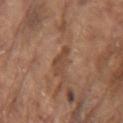Findings:
- notes — catalogued during a skin exam; not biopsied
- body site — the left upper arm
- diameter — ≈3.5 mm
- automated metrics — border irregularity of about 3.5 on a 0–10 scale, internal color variation of about 1.5 on a 0–10 scale, and peripheral color asymmetry of about 0.5; a lesion-detection confidence of about 100/100
- image source — 15 mm crop, total-body photography
- patient — male, in their 80s
- lighting — white-light illumination